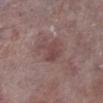Part of a total-body skin-imaging series; this lesion was reviewed on a skin check and was not flagged for biopsy. The patient is a male roughly 75 years of age. A roughly 15 mm field-of-view crop from a total-body skin photograph. Located on the left lower leg.